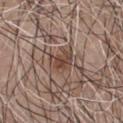biopsy status: total-body-photography surveillance lesion; no biopsy
imaging modality: ~15 mm tile from a whole-body skin photo
illumination: white-light
size: ~3 mm (longest diameter)
TBP lesion metrics: a lesion area of about 6.5 mm² and a shape eccentricity near 0.6; roughly 9 lightness units darker than nearby skin and a normalized border contrast of about 7.5; a border-irregularity index near 4/10 and internal color variation of about 3.5 on a 0–10 scale; a nevus-likeness score of about 5/100
anatomic site: the chest
patient: male, roughly 75 years of age